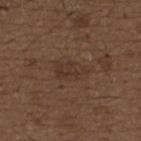The lesion was tiled from a total-body skin photograph and was not biopsied. About 4.5 mm across. A lesion tile, about 15 mm wide, cut from a 3D total-body photograph. Captured under white-light illumination. Located on the upper back. Automated image analysis of the tile measured a lesion color around L≈34 a*≈15 b*≈24 in CIELAB, about 5 CIELAB-L* units darker than the surrounding skin, and a normalized border contrast of about 5. The software also gave an automated nevus-likeness rating near 0 out of 100 and a detector confidence of about 95 out of 100 that the crop contains a lesion. A male patient aged around 50.